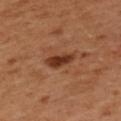{"biopsy_status": "not biopsied; imaged during a skin examination", "patient": {"sex": "male", "age_approx": 50}, "site": "upper back", "image": {"source": "total-body photography crop", "field_of_view_mm": 15}, "lighting": "cross-polarized"}